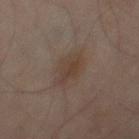Recorded during total-body skin imaging; not selected for excision or biopsy.
Longest diameter approximately 4 mm.
Cropped from a whole-body photographic skin survey; the tile spans about 15 mm.
On the right thigh.
The tile uses cross-polarized illumination.
The lesion-visualizer software estimated a shape eccentricity near 0.6. The analysis additionally found a lesion–skin lightness drop of about 6 and a lesion-to-skin contrast of about 6 (normalized; higher = more distinct). The analysis additionally found a classifier nevus-likeness of about 35/100 and a detector confidence of about 100 out of 100 that the crop contains a lesion.
The subject is a male aged around 65.